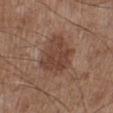The lesion was tiled from a total-body skin photograph and was not biopsied. A 15 mm close-up extracted from a 3D total-body photography capture. The patient is a male aged 53 to 57. The tile uses white-light illumination. The total-body-photography lesion software estimated a lesion area of about 17 mm², a shape eccentricity near 0.4, and a shape-asymmetry score of about 0.25 (0 = symmetric). The analysis additionally found an average lesion color of about L≈42 a*≈19 b*≈26 (CIELAB), about 9 CIELAB-L* units darker than the surrounding skin, and a normalized border contrast of about 7. The analysis additionally found a nevus-likeness score of about 15/100. The lesion's longest dimension is about 5 mm. The lesion is located on the left lower leg.